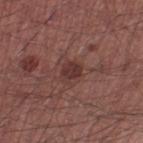workup = total-body-photography surveillance lesion; no biopsy.